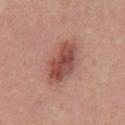The lesion was photographed on a routine skin check and not biopsied; there is no pathology result. An algorithmic analysis of the crop reported a border-irregularity index near 2/10 and radial color variation of about 1.5. The patient is a male in their mid-50s. Cropped from a total-body skin-imaging series; the visible field is about 15 mm. On the chest. Longest diameter approximately 6 mm.